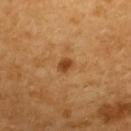  biopsy_status: not biopsied; imaged during a skin examination
  image:
    source: total-body photography crop
    field_of_view_mm: 15
  lighting: cross-polarized
  site: upper back
  patient:
    sex: female
    age_approx: 55
  automated_metrics:
    area_mm2_approx: 3.0
    eccentricity: 0.6
    cielab_L: 46
    cielab_a: 25
    cielab_b: 42
    vs_skin_darker_L: 11.0
    vs_skin_contrast_norm: 8.5
  lesion_size:
    long_diameter_mm_approx: 2.0